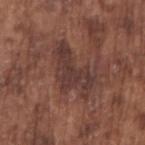• biopsy status — imaged on a skin check; not biopsied
• diameter — ≈6 mm
• lighting — white-light illumination
• imaging modality — total-body-photography crop, ~15 mm field of view
• subject — male, approximately 75 years of age
• body site — the right upper arm
• automated metrics — an area of roughly 16 mm² and a symmetry-axis asymmetry near 0.5